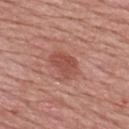follow-up=total-body-photography surveillance lesion; no biopsy
anatomic site=the upper back
patient=male, aged 48 to 52
size=≈3 mm
acquisition=15 mm crop, total-body photography